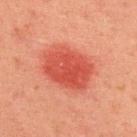No biopsy was performed on this lesion — it was imaged during a full skin examination and was not determined to be concerning. A roughly 15 mm field-of-view crop from a total-body skin photograph. On the upper back. A male patient, in their mid-40s.